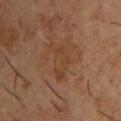Part of a total-body skin-imaging series; this lesion was reviewed on a skin check and was not flagged for biopsy. Captured under cross-polarized illumination. The lesion's longest dimension is about 5 mm. The lesion is on the chest. Cropped from a whole-body photographic skin survey; the tile spans about 15 mm. An algorithmic analysis of the crop reported a border-irregularity index near 5.5/10, a within-lesion color-variation index near 2.5/10, and peripheral color asymmetry of about 0.5. The analysis additionally found an automated nevus-likeness rating near 0 out of 100 and a detector confidence of about 100 out of 100 that the crop contains a lesion. The subject is a male approximately 55 years of age.